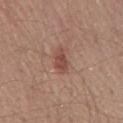{"biopsy_status": "not biopsied; imaged during a skin examination", "automated_metrics": {"area_mm2_approx": 4.5, "eccentricity": 0.85, "shape_asymmetry": 0.25, "border_irregularity_0_10": 2.5, "peripheral_color_asymmetry": 0.5, "nevus_likeness_0_100": 65, "lesion_detection_confidence_0_100": 100}, "lesion_size": {"long_diameter_mm_approx": 3.0}, "site": "chest", "image": {"source": "total-body photography crop", "field_of_view_mm": 15}, "patient": {"sex": "male", "age_approx": 55}, "lighting": "white-light"}